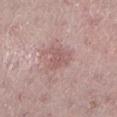This lesion was catalogued during total-body skin photography and was not selected for biopsy. The patient is a female aged approximately 45. On the left lower leg. This image is a 15 mm lesion crop taken from a total-body photograph. Measured at roughly 3 mm in maximum diameter.